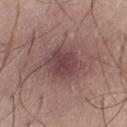biopsy status=imaged on a skin check; not biopsied | patient=male, in their 50s | anatomic site=the left thigh | tile lighting=white-light | lesion diameter=~5 mm (longest diameter) | image=15 mm crop, total-body photography.